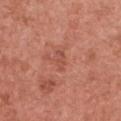Notes:
* workup — catalogued during a skin exam; not biopsied
* lesion size — ~2.5 mm (longest diameter)
* imaging modality — total-body-photography crop, ~15 mm field of view
* patient — female, aged around 50
* anatomic site — the chest
* illumination — white-light illumination